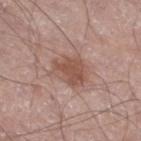follow-up: no biopsy performed (imaged during a skin exam)